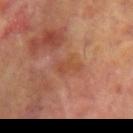biopsy status: no biopsy performed (imaged during a skin exam) | subject: male, roughly 65 years of age | automated lesion analysis: an area of roughly 4.5 mm², an outline eccentricity of about 0.9 (0 = round, 1 = elongated), and two-axis asymmetry of about 0.45; a lesion color around L≈47 a*≈24 b*≈32 in CIELAB and about 6 CIELAB-L* units darker than the surrounding skin; internal color variation of about 2 on a 0–10 scale and peripheral color asymmetry of about 0.5 | acquisition: total-body-photography crop, ~15 mm field of view | lesion size: ≈3.5 mm | site: the left upper arm.